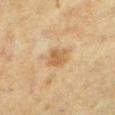The lesion is on the left lower leg.
The tile uses cross-polarized illumination.
Cropped from a whole-body photographic skin survey; the tile spans about 15 mm.
The recorded lesion diameter is about 2.5 mm.
A female patient aged 53 to 57.
An algorithmic analysis of the crop reported a within-lesion color-variation index near 2.5/10 and radial color variation of about 1.